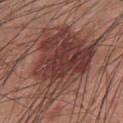notes: catalogued during a skin exam; not biopsied
subject: male, aged around 60
acquisition: ~15 mm crop, total-body skin-cancer survey
size: about 10.5 mm
tile lighting: white-light
body site: the front of the torso